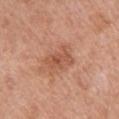Part of a total-body skin-imaging series; this lesion was reviewed on a skin check and was not flagged for biopsy. A female patient aged approximately 40. From the arm. A roughly 15 mm field-of-view crop from a total-body skin photograph.